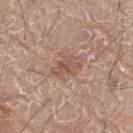Findings:
* biopsy status: catalogued during a skin exam; not biopsied
* diameter: ≈3.5 mm
* subject: male, about 80 years old
* lighting: white-light illumination
* automated metrics: a footprint of about 7.5 mm², a shape eccentricity near 0.75, and two-axis asymmetry of about 0.25; a border-irregularity index near 3/10, internal color variation of about 4 on a 0–10 scale, and a peripheral color-asymmetry measure near 1
* acquisition: total-body-photography crop, ~15 mm field of view
* body site: the left leg